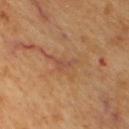<tbp_lesion>
  <biopsy_status>not biopsied; imaged during a skin examination</biopsy_status>
  <site>upper back</site>
  <image>
    <source>total-body photography crop</source>
    <field_of_view_mm>15</field_of_view_mm>
  </image>
  <patient>
    <age_approx>60</age_approx>
  </patient>
</tbp_lesion>